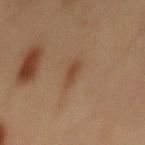Imaged during a routine full-body skin examination; the lesion was not biopsied and no histopathology is available. From the abdomen. A 15 mm crop from a total-body photograph taken for skin-cancer surveillance. About 3 mm across. A male subject, about 70 years old. The tile uses cross-polarized illumination. Automated image analysis of the tile measured a footprint of about 3.5 mm², a shape eccentricity near 0.85, and two-axis asymmetry of about 0.2. It also reported a border-irregularity index near 2/10, a within-lesion color-variation index near 1/10, and peripheral color asymmetry of about 0.5.